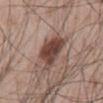{
  "biopsy_status": "not biopsied; imaged during a skin examination",
  "lesion_size": {
    "long_diameter_mm_approx": 5.0
  },
  "site": "front of the torso",
  "patient": {
    "sex": "male",
    "age_approx": 55
  },
  "image": {
    "source": "total-body photography crop",
    "field_of_view_mm": 15
  },
  "automated_metrics": {
    "border_irregularity_0_10": 3.5,
    "color_variation_0_10": 4.5,
    "peripheral_color_asymmetry": 1.5
  }
}